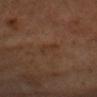Q: Was this lesion biopsied?
A: catalogued during a skin exam; not biopsied
Q: Patient demographics?
A: male, in their mid- to late 40s
Q: What is the anatomic site?
A: the head or neck
Q: What kind of image is this?
A: ~15 mm crop, total-body skin-cancer survey